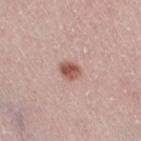{
  "biopsy_status": "not biopsied; imaged during a skin examination",
  "image": {
    "source": "total-body photography crop",
    "field_of_view_mm": 15
  },
  "lesion_size": {
    "long_diameter_mm_approx": 2.5
  },
  "site": "right thigh",
  "automated_metrics": {
    "area_mm2_approx": 4.0,
    "eccentricity": 0.55,
    "shape_asymmetry": 0.15,
    "nevus_likeness_0_100": 95,
    "lesion_detection_confidence_0_100": 100
  },
  "patient": {
    "sex": "female",
    "age_approx": 40
  }
}